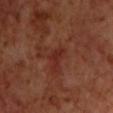| feature | finding |
|---|---|
| follow-up | total-body-photography surveillance lesion; no biopsy |
| body site | the chest |
| image-analysis metrics | a footprint of about 3.5 mm², an outline eccentricity of about 0.65 (0 = round, 1 = elongated), and a symmetry-axis asymmetry near 0.55; a border-irregularity index near 5/10 and a color-variation rating of about 0.5/10 |
| image source | total-body-photography crop, ~15 mm field of view |
| patient | male, aged around 70 |
| lesion diameter | ≈2.5 mm |
| lighting | cross-polarized illumination |